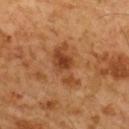This image is a 15 mm lesion crop taken from a total-body photograph. The subject is a male approximately 60 years of age. Measured at roughly 5 mm in maximum diameter. The total-body-photography lesion software estimated a lesion area of about 10 mm², an eccentricity of roughly 0.85, and a shape-asymmetry score of about 0.4 (0 = symmetric). And it measured internal color variation of about 4 on a 0–10 scale and radial color variation of about 1. It also reported an automated nevus-likeness rating near 70 out of 100 and a detector confidence of about 100 out of 100 that the crop contains a lesion. The lesion is located on the upper back.